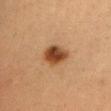biopsy status — imaged on a skin check; not biopsied
diameter — ≈3.5 mm
location — the head or neck
patient — female, aged 38–42
acquisition — ~15 mm tile from a whole-body skin photo
TBP lesion metrics — a lesion area of about 8.5 mm², an outline eccentricity of about 0.6 (0 = round, 1 = elongated), and a symmetry-axis asymmetry near 0.15; an average lesion color of about L≈40 a*≈20 b*≈32 (CIELAB); lesion-presence confidence of about 100/100
lighting — cross-polarized